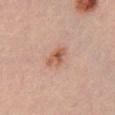The lesion was tiled from a total-body skin photograph and was not biopsied.
A lesion tile, about 15 mm wide, cut from a 3D total-body photograph.
A female subject, roughly 25 years of age.
Automated tile analysis of the lesion measured a shape eccentricity near 0.8 and two-axis asymmetry of about 0.25. It also reported a border-irregularity rating of about 3/10, a within-lesion color-variation index near 5.5/10, and radial color variation of about 2.
This is a white-light tile.
On the front of the torso.
About 3.5 mm across.